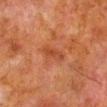Part of a total-body skin-imaging series; this lesion was reviewed on a skin check and was not flagged for biopsy. A male subject, about 80 years old. The lesion-visualizer software estimated a lesion area of about 5 mm², an outline eccentricity of about 0.9 (0 = round, 1 = elongated), and two-axis asymmetry of about 0.25. It also reported a lesion color around L≈37 a*≈24 b*≈31 in CIELAB and about 6 CIELAB-L* units darker than the surrounding skin. And it measured a border-irregularity rating of about 3/10, internal color variation of about 3 on a 0–10 scale, and radial color variation of about 1. A close-up tile cropped from a whole-body skin photograph, about 15 mm across. The lesion's longest dimension is about 3.5 mm. Located on the right lower leg.